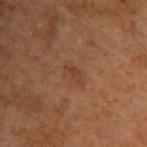biopsy status=catalogued during a skin exam; not biopsied
patient=male, roughly 70 years of age
site=the upper back
image=~15 mm crop, total-body skin-cancer survey